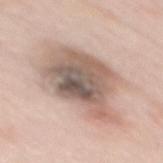Findings:
• follow-up — total-body-photography surveillance lesion; no biopsy
• TBP lesion metrics — a footprint of about 38 mm², an eccentricity of roughly 0.85, and a shape-asymmetry score of about 0.3 (0 = symmetric); a classifier nevus-likeness of about 0/100 and a lesion-detection confidence of about 100/100
• image — total-body-photography crop, ~15 mm field of view
• patient — female, aged 63 to 67
• diameter — about 10 mm
• location — the mid back